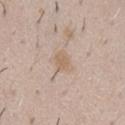{
  "biopsy_status": "not biopsied; imaged during a skin examination",
  "patient": {
    "sex": "male",
    "age_approx": 50
  },
  "image": {
    "source": "total-body photography crop",
    "field_of_view_mm": 15
  },
  "site": "chest"
}